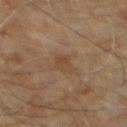Clinical impression:
Part of a total-body skin-imaging series; this lesion was reviewed on a skin check and was not flagged for biopsy.
Image and clinical context:
The lesion is located on the front of the torso. A 15 mm close-up extracted from a 3D total-body photography capture. This is a cross-polarized tile. About 2.5 mm across. A male patient, aged 58–62.